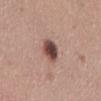notes — catalogued during a skin exam; not biopsied | location — the leg | patient — female, aged 28 to 32 | lesion size — about 2.5 mm | automated metrics — a lesion area of about 6 mm², an outline eccentricity of about 0.5 (0 = round, 1 = elongated), and a symmetry-axis asymmetry near 0.15; about 18 CIELAB-L* units darker than the surrounding skin and a lesion-to-skin contrast of about 12.5 (normalized; higher = more distinct); border irregularity of about 1.5 on a 0–10 scale and a peripheral color-asymmetry measure near 1.5; a classifier nevus-likeness of about 95/100 | image source — ~15 mm tile from a whole-body skin photo.